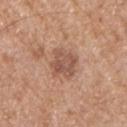<lesion>
  <biopsy_status>not biopsied; imaged during a skin examination</biopsy_status>
  <site>chest</site>
  <image>
    <source>total-body photography crop</source>
    <field_of_view_mm>15</field_of_view_mm>
  </image>
  <lighting>white-light</lighting>
  <patient>
    <sex>male</sex>
    <age_approx>65</age_approx>
  </patient>
  <automated_metrics>
    <vs_skin_contrast_norm>7.0</vs_skin_contrast_norm>
    <border_irregularity_0_10>2.5</border_irregularity_0_10>
    <color_variation_0_10>3.5</color_variation_0_10>
    <peripheral_color_asymmetry>1.0</peripheral_color_asymmetry>
    <nevus_likeness_0_100>0</nevus_likeness_0_100>
    <lesion_detection_confidence_0_100>100</lesion_detection_confidence_0_100>
  </automated_metrics>
  <lesion_size>
    <long_diameter_mm_approx>3.5</long_diameter_mm_approx>
  </lesion_size>
</lesion>